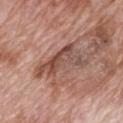Case summary:
* tile lighting: white-light illumination
* subject: male, roughly 70 years of age
* location: the mid back
* acquisition: 15 mm crop, total-body photography